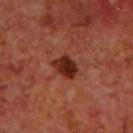The lesion was photographed on a routine skin check and not biopsied; there is no pathology result. The lesion is located on the upper back. Cropped from a whole-body photographic skin survey; the tile spans about 15 mm. The total-body-photography lesion software estimated an eccentricity of roughly 0.7 and a symmetry-axis asymmetry near 0.2. It also reported a border-irregularity rating of about 2.5/10. The tile uses cross-polarized illumination. A male subject, aged 68 to 72.